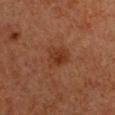Q: Was this lesion biopsied?
A: total-body-photography surveillance lesion; no biopsy
Q: Who is the patient?
A: female, roughly 40 years of age
Q: Automated lesion metrics?
A: a lesion color around L≈29 a*≈20 b*≈28 in CIELAB, about 6 CIELAB-L* units darker than the surrounding skin, and a lesion-to-skin contrast of about 6.5 (normalized; higher = more distinct); lesion-presence confidence of about 100/100
Q: Lesion location?
A: the front of the torso
Q: What is the lesion's diameter?
A: about 3.5 mm
Q: What is the imaging modality?
A: ~15 mm tile from a whole-body skin photo